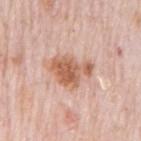location=the mid back
patient=male, approximately 80 years of age
size=~5 mm (longest diameter)
lighting=white-light
imaging modality=15 mm crop, total-body photography